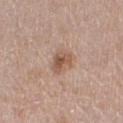Impression: The lesion was tiled from a total-body skin photograph and was not biopsied. Acquisition and patient details: A female subject, aged approximately 60. From the right thigh. Cropped from a total-body skin-imaging series; the visible field is about 15 mm.